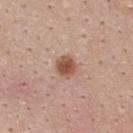- notes — total-body-photography surveillance lesion; no biopsy
- image source — ~15 mm tile from a whole-body skin photo
- automated lesion analysis — a lesion area of about 5 mm², an outline eccentricity of about 0.35 (0 = round, 1 = elongated), and a shape-asymmetry score of about 0.1 (0 = symmetric); a mean CIELAB color near L≈52 a*≈22 b*≈29, a lesion–skin lightness drop of about 13, and a normalized border contrast of about 9.5; a border-irregularity rating of about 1/10, a within-lesion color-variation index near 3.5/10, and radial color variation of about 1.5
- lighting — white-light
- body site — the upper back
- patient — female, about 25 years old